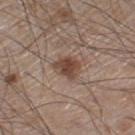Assessment:
No biopsy was performed on this lesion — it was imaged during a full skin examination and was not determined to be concerning.
Context:
Located on the right lower leg. Longest diameter approximately 3 mm. This image is a 15 mm lesion crop taken from a total-body photograph. A male subject aged approximately 60.